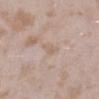  biopsy_status: not biopsied; imaged during a skin examination
  image:
    source: total-body photography crop
    field_of_view_mm: 15
  site: left lower leg
  lesion_size:
    long_diameter_mm_approx: 2.5
  patient:
    sex: female
    age_approx: 25
  lighting: white-light